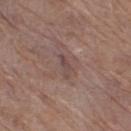Notes:
* notes: total-body-photography surveillance lesion; no biopsy
* body site: the right thigh
* diameter: ~3 mm (longest diameter)
* imaging modality: ~15 mm tile from a whole-body skin photo
* subject: female, aged around 70